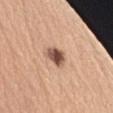Recorded during total-body skin imaging; not selected for excision or biopsy. The tile uses white-light illumination. A female subject, approximately 60 years of age. The lesion is located on the left upper arm. The lesion-visualizer software estimated a lesion area of about 5 mm² and a shape eccentricity near 0.35. It also reported border irregularity of about 2.5 on a 0–10 scale. And it measured a nevus-likeness score of about 95/100 and a detector confidence of about 100 out of 100 that the crop contains a lesion. Cropped from a total-body skin-imaging series; the visible field is about 15 mm.